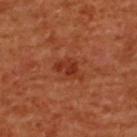Impression:
Part of a total-body skin-imaging series; this lesion was reviewed on a skin check and was not flagged for biopsy.
Clinical summary:
Captured under cross-polarized illumination. The subject is a female aged 53–57. Measured at roughly 3.5 mm in maximum diameter. A 15 mm close-up extracted from a 3D total-body photography capture. Located on the upper back.